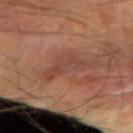• imaging modality: total-body-photography crop, ~15 mm field of view
• subject: male, in their 60s
• body site: the arm